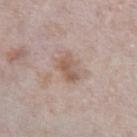| feature | finding |
|---|---|
| notes | catalogued during a skin exam; not biopsied |
| patient | male, aged around 60 |
| location | the abdomen |
| image source | total-body-photography crop, ~15 mm field of view |